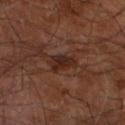Q: Was this lesion biopsied?
A: no biopsy performed (imaged during a skin exam)
Q: What are the patient's age and sex?
A: male, aged approximately 60
Q: What lighting was used for the tile?
A: cross-polarized
Q: Lesion location?
A: the right leg
Q: How was this image acquired?
A: 15 mm crop, total-body photography